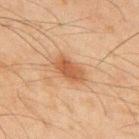This lesion was catalogued during total-body skin photography and was not selected for biopsy.
Approximately 4 mm at its widest.
The lesion is on the upper back.
This is a cross-polarized tile.
A male subject about 45 years old.
A close-up tile cropped from a whole-body skin photograph, about 15 mm across.
An algorithmic analysis of the crop reported a footprint of about 7 mm² and a symmetry-axis asymmetry near 0.2. And it measured an average lesion color of about L≈46 a*≈20 b*≈32 (CIELAB), roughly 10 lightness units darker than nearby skin, and a lesion-to-skin contrast of about 7.5 (normalized; higher = more distinct). And it measured a border-irregularity rating of about 2.5/10, a color-variation rating of about 3/10, and a peripheral color-asymmetry measure near 1. It also reported a nevus-likeness score of about 95/100 and a detector confidence of about 100 out of 100 that the crop contains a lesion.